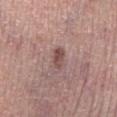follow-up: catalogued during a skin exam; not biopsied | lesion diameter: ~3 mm (longest diameter) | tile lighting: white-light | automated metrics: an average lesion color of about L≈49 a*≈20 b*≈21 (CIELAB) and a lesion-to-skin contrast of about 7.5 (normalized; higher = more distinct); a border-irregularity rating of about 2.5/10, a color-variation rating of about 3.5/10, and a peripheral color-asymmetry measure near 1.5 | subject: male, about 65 years old | image: 15 mm crop, total-body photography | anatomic site: the left lower leg.